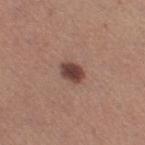Case summary:
- biopsy status — imaged on a skin check; not biopsied
- image source — total-body-photography crop, ~15 mm field of view
- patient — female, roughly 30 years of age
- TBP lesion metrics — a mean CIELAB color near L≈42 a*≈20 b*≈23, a lesion–skin lightness drop of about 14, and a lesion-to-skin contrast of about 11 (normalized; higher = more distinct); a border-irregularity index near 1.5/10, a within-lesion color-variation index near 3.5/10, and peripheral color asymmetry of about 1; a nevus-likeness score of about 95/100 and lesion-presence confidence of about 100/100
- site — the right thigh
- illumination — white-light